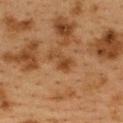Q: Was this lesion biopsied?
A: catalogued during a skin exam; not biopsied
Q: How was this image acquired?
A: total-body-photography crop, ~15 mm field of view
Q: Lesion location?
A: the back
Q: Lesion size?
A: ~3 mm (longest diameter)
Q: Illumination type?
A: cross-polarized illumination
Q: Patient demographics?
A: female, roughly 40 years of age
Q: What did automated image analysis measure?
A: an area of roughly 4.5 mm², an eccentricity of roughly 0.85, and a shape-asymmetry score of about 0.4 (0 = symmetric); an average lesion color of about L≈37 a*≈19 b*≈32 (CIELAB), a lesion–skin lightness drop of about 7, and a normalized border contrast of about 7